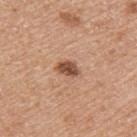Assessment:
This lesion was catalogued during total-body skin photography and was not selected for biopsy.
Context:
A female subject, aged around 70. The lesion is on the left upper arm. Measured at roughly 3 mm in maximum diameter. The total-body-photography lesion software estimated a mean CIELAB color near L≈50 a*≈23 b*≈31, a lesion–skin lightness drop of about 15, and a lesion-to-skin contrast of about 10.5 (normalized; higher = more distinct). A 15 mm close-up extracted from a 3D total-body photography capture.